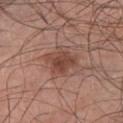tile lighting = white-light
location = the chest
patient = male, approximately 30 years of age
imaging modality = ~15 mm crop, total-body skin-cancer survey